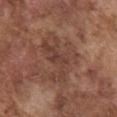This lesion was catalogued during total-body skin photography and was not selected for biopsy. A 15 mm close-up tile from a total-body photography series done for melanoma screening. On the chest. The patient is a male aged around 75.